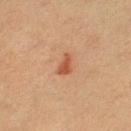Findings:
- acquisition: total-body-photography crop, ~15 mm field of view
- TBP lesion metrics: a footprint of about 3.5 mm², a shape eccentricity near 0.8, and two-axis asymmetry of about 0.4; a border-irregularity index near 3.5/10 and a peripheral color-asymmetry measure near 0.5; an automated nevus-likeness rating near 95 out of 100 and a lesion-detection confidence of about 100/100
- patient: female, aged 38 to 42
- anatomic site: the left lower leg
- diameter: ~2.5 mm (longest diameter)